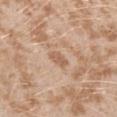– biopsy status: total-body-photography surveillance lesion; no biopsy
– image source: ~15 mm tile from a whole-body skin photo
– subject: female, approximately 25 years of age
– TBP lesion metrics: an area of roughly 3.5 mm², an outline eccentricity of about 0.8 (0 = round, 1 = elongated), and a shape-asymmetry score of about 0.15 (0 = symmetric); a nevus-likeness score of about 0/100 and a detector confidence of about 100 out of 100 that the crop contains a lesion
– site: the left upper arm